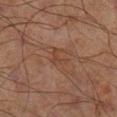{
  "biopsy_status": "not biopsied; imaged during a skin examination",
  "lesion_size": {
    "long_diameter_mm_approx": 2.5
  },
  "lighting": "cross-polarized",
  "image": {
    "source": "total-body photography crop",
    "field_of_view_mm": 15
  },
  "patient": {
    "sex": "male",
    "age_approx": 70
  },
  "site": "right lower leg"
}